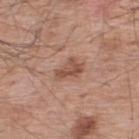notes: total-body-photography surveillance lesion; no biopsy
automated metrics: a footprint of about 5 mm², an eccentricity of roughly 0.85, and a symmetry-axis asymmetry near 0.45; a lesion–skin lightness drop of about 10 and a lesion-to-skin contrast of about 7 (normalized; higher = more distinct); a border-irregularity index near 4.5/10, a within-lesion color-variation index near 2.5/10, and peripheral color asymmetry of about 1; a classifier nevus-likeness of about 35/100 and a detector confidence of about 100 out of 100 that the crop contains a lesion
acquisition: ~15 mm crop, total-body skin-cancer survey
anatomic site: the upper back
patient: male, aged 53–57
lesion size: about 3.5 mm